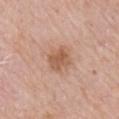Imaged during a routine full-body skin examination; the lesion was not biopsied and no histopathology is available.
From the arm.
The subject is a male aged 58 to 62.
Cropped from a total-body skin-imaging series; the visible field is about 15 mm.